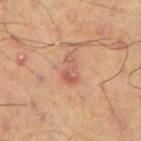Imaged during a routine full-body skin examination; the lesion was not biopsied and no histopathology is available. A male patient in their 70s. Automated image analysis of the tile measured a footprint of about 4.5 mm², an eccentricity of roughly 0.9, and two-axis asymmetry of about 0.35. It also reported a lesion color around L≈46 a*≈21 b*≈25 in CIELAB, roughly 7 lightness units darker than nearby skin, and a lesion-to-skin contrast of about 6 (normalized; higher = more distinct). Cropped from a total-body skin-imaging series; the visible field is about 15 mm. Imaged with cross-polarized lighting. The lesion is on the arm.